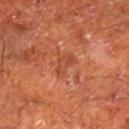{"biopsy_status": "not biopsied; imaged during a skin examination", "automated_metrics": {"area_mm2_approx": 3.5, "cielab_L": 45, "cielab_a": 29, "cielab_b": 35, "vs_skin_darker_L": 7.0, "nevus_likeness_0_100": 0}, "lighting": "cross-polarized", "site": "right lower leg", "lesion_size": {"long_diameter_mm_approx": 3.0}, "patient": {"sex": "male", "age_approx": 65}, "image": {"source": "total-body photography crop", "field_of_view_mm": 15}}